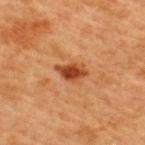Recorded during total-body skin imaging; not selected for excision or biopsy. Longest diameter approximately 3.5 mm. From the upper back. Captured under cross-polarized illumination. A female subject in their 40s. An algorithmic analysis of the crop reported an area of roughly 5 mm², an outline eccentricity of about 0.8 (0 = round, 1 = elongated), and a symmetry-axis asymmetry near 0.4. And it measured a lesion color around L≈39 a*≈27 b*≈36 in CIELAB and a normalized border contrast of about 10.5. The software also gave a lesion-detection confidence of about 100/100. Cropped from a total-body skin-imaging series; the visible field is about 15 mm.